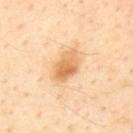Q: Was this lesion biopsied?
A: catalogued during a skin exam; not biopsied
Q: Who is the patient?
A: male, in their mid- to late 50s
Q: Where on the body is the lesion?
A: the upper back
Q: What kind of image is this?
A: ~15 mm crop, total-body skin-cancer survey
Q: How was the tile lit?
A: cross-polarized